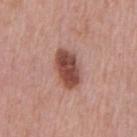follow-up = total-body-photography surveillance lesion; no biopsy | TBP lesion metrics = a nevus-likeness score of about 95/100 and a detector confidence of about 100 out of 100 that the crop contains a lesion | imaging modality = ~15 mm tile from a whole-body skin photo | patient = male, aged 68–72 | lesion diameter = ~4.5 mm (longest diameter) | illumination = white-light | body site = the front of the torso.